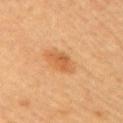Clinical impression: Part of a total-body skin-imaging series; this lesion was reviewed on a skin check and was not flagged for biopsy. Clinical summary: A 15 mm close-up extracted from a 3D total-body photography capture. Approximately 3.5 mm at its widest. From the right upper arm. A female subject roughly 60 years of age. The tile uses cross-polarized illumination.